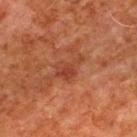The lesion-visualizer software estimated a mean CIELAB color near L≈33 a*≈24 b*≈27 and roughly 6 lightness units darker than nearby skin. The analysis additionally found a nevus-likeness score of about 0/100.
This is a cross-polarized tile.
Longest diameter approximately 4 mm.
On the upper back.
Cropped from a total-body skin-imaging series; the visible field is about 15 mm.
The subject is a male about 80 years old.